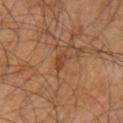Q: Is there a histopathology result?
A: imaged on a skin check; not biopsied
Q: What is the imaging modality?
A: 15 mm crop, total-body photography
Q: What lighting was used for the tile?
A: cross-polarized
Q: Patient demographics?
A: male, aged 58–62
Q: What did automated image analysis measure?
A: a border-irregularity index near 2/10, a within-lesion color-variation index near 1.5/10, and a peripheral color-asymmetry measure near 0.5; a nevus-likeness score of about 0/100
Q: Where on the body is the lesion?
A: the left upper arm
Q: Lesion size?
A: ≈3 mm